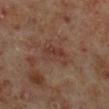Assessment: Recorded during total-body skin imaging; not selected for excision or biopsy. Context: On the right lower leg. About 4 mm across. A roughly 15 mm field-of-view crop from a total-body skin photograph. The patient is a male about 60 years old. An algorithmic analysis of the crop reported a classifier nevus-likeness of about 0/100 and lesion-presence confidence of about 100/100.